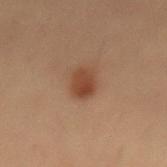Clinical impression:
Part of a total-body skin-imaging series; this lesion was reviewed on a skin check and was not flagged for biopsy.
Clinical summary:
The patient is a male aged 53–57. The tile uses cross-polarized illumination. Located on the lower back. Cropped from a whole-body photographic skin survey; the tile spans about 15 mm. An algorithmic analysis of the crop reported an eccentricity of roughly 0.8 and two-axis asymmetry of about 0.15. The analysis additionally found a classifier nevus-likeness of about 95/100 and lesion-presence confidence of about 100/100.